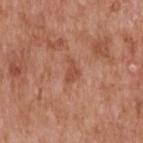Assessment: Imaged during a routine full-body skin examination; the lesion was not biopsied and no histopathology is available. Clinical summary: Approximately 2.5 mm at its widest. A male patient, in their 60s. An algorithmic analysis of the crop reported an average lesion color of about L≈50 a*≈26 b*≈32 (CIELAB), roughly 8 lightness units darker than nearby skin, and a lesion-to-skin contrast of about 6 (normalized; higher = more distinct). The software also gave a detector confidence of about 100 out of 100 that the crop contains a lesion. A 15 mm close-up tile from a total-body photography series done for melanoma screening. Captured under white-light illumination. Located on the upper back.